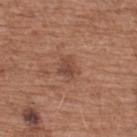- biopsy status — imaged on a skin check; not biopsied
- lesion size — ≈2.5 mm
- automated lesion analysis — a border-irregularity rating of about 3.5/10, a within-lesion color-variation index near 2.5/10, and radial color variation of about 1; an automated nevus-likeness rating near 15 out of 100 and lesion-presence confidence of about 100/100
- imaging modality — ~15 mm tile from a whole-body skin photo
- site — the upper back
- patient — male, aged approximately 75
- tile lighting — white-light illumination